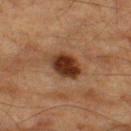Impression: Recorded during total-body skin imaging; not selected for excision or biopsy. Clinical summary: Captured under cross-polarized illumination. A 15 mm close-up extracted from a 3D total-body photography capture. The lesion is on the left thigh. A male patient, aged approximately 85 or older. The total-body-photography lesion software estimated a border-irregularity index near 1.5/10, internal color variation of about 5.5 on a 0–10 scale, and radial color variation of about 1.5. About 4.5 mm across.